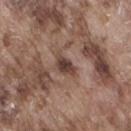notes = total-body-photography surveillance lesion; no biopsy
image = ~15 mm tile from a whole-body skin photo
subject = male, aged 73–77
body site = the right thigh